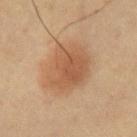Clinical impression: The lesion was tiled from a total-body skin photograph and was not biopsied. Acquisition and patient details: A 15 mm close-up tile from a total-body photography series done for melanoma screening. The tile uses cross-polarized illumination. A male subject, aged approximately 60. Measured at roughly 6 mm in maximum diameter. The lesion is on the left upper arm. The lesion-visualizer software estimated a footprint of about 21 mm², an outline eccentricity of about 0.65 (0 = round, 1 = elongated), and two-axis asymmetry of about 0.15. It also reported an automated nevus-likeness rating near 95 out of 100 and a lesion-detection confidence of about 100/100.